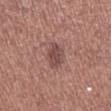Captured during whole-body skin photography for melanoma surveillance; the lesion was not biopsied.
The subject is a male approximately 70 years of age.
A lesion tile, about 15 mm wide, cut from a 3D total-body photograph.
The recorded lesion diameter is about 3.5 mm.
On the right lower leg.
The tile uses white-light illumination.